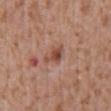follow-up: no biopsy performed (imaged during a skin exam)
subject: male, approximately 45 years of age
TBP lesion metrics: a footprint of about 4.5 mm², a shape eccentricity near 0.7, and two-axis asymmetry of about 0.35; a lesion color around L≈48 a*≈23 b*≈28 in CIELAB, a lesion–skin lightness drop of about 10, and a normalized lesion–skin contrast near 7.5; a detector confidence of about 100 out of 100 that the crop contains a lesion
location: the front of the torso
acquisition: ~15 mm crop, total-body skin-cancer survey
size: about 3 mm
lighting: white-light illumination